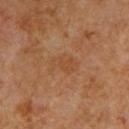Q: Was this lesion biopsied?
A: catalogued during a skin exam; not biopsied
Q: Automated lesion metrics?
A: a border-irregularity index near 3.5/10, a color-variation rating of about 2.5/10, and radial color variation of about 1
Q: What are the patient's age and sex?
A: male, aged 58 to 62
Q: What is the lesion's diameter?
A: ~3.5 mm (longest diameter)
Q: What kind of image is this?
A: ~15 mm tile from a whole-body skin photo
Q: Lesion location?
A: the chest